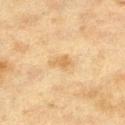Impression:
No biopsy was performed on this lesion — it was imaged during a full skin examination and was not determined to be concerning.
Image and clinical context:
The lesion is on the leg. A roughly 15 mm field-of-view crop from a total-body skin photograph. The patient is a female roughly 40 years of age.